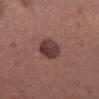{"biopsy_status": "not biopsied; imaged during a skin examination", "site": "right thigh", "patient": {"sex": "female", "age_approx": 40}, "lesion_size": {"long_diameter_mm_approx": 4.5}, "lighting": "white-light", "image": {"source": "total-body photography crop", "field_of_view_mm": 15}}